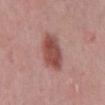Case summary:
- patient — male, about 50 years old
- anatomic site — the chest
- acquisition — total-body-photography crop, ~15 mm field of view
- diameter — about 5 mm